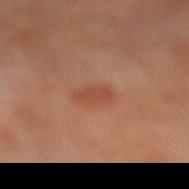Assessment:
The lesion was tiled from a total-body skin photograph and was not biopsied.
Acquisition and patient details:
Approximately 3 mm at its widest. The subject is a male approximately 65 years of age. A roughly 15 mm field-of-view crop from a total-body skin photograph. Imaged with cross-polarized lighting. On the left lower leg.